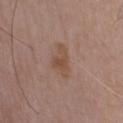<tbp_lesion>
  <biopsy_status>not biopsied; imaged during a skin examination</biopsy_status>
  <lighting>white-light</lighting>
  <image>
    <source>total-body photography crop</source>
    <field_of_view_mm>15</field_of_view_mm>
  </image>
  <site>front of the torso</site>
  <patient>
    <sex>male</sex>
    <age_approx>75</age_approx>
  </patient>
</tbp_lesion>